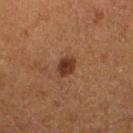workup — catalogued during a skin exam; not biopsied
image — ~15 mm tile from a whole-body skin photo
subject — female, in their 50s
illumination — cross-polarized
automated metrics — two-axis asymmetry of about 0.25; an average lesion color of about L≈28 a*≈19 b*≈25 (CIELAB), about 10 CIELAB-L* units darker than the surrounding skin, and a normalized lesion–skin contrast near 10; a within-lesion color-variation index near 2/10 and peripheral color asymmetry of about 0.5; a nevus-likeness score of about 100/100 and lesion-presence confidence of about 100/100
lesion diameter — about 2.5 mm
site — the left lower leg